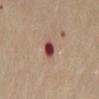This lesion was catalogued during total-body skin photography and was not selected for biopsy. A region of skin cropped from a whole-body photographic capture, roughly 15 mm wide. Automated image analysis of the tile measured a lesion-detection confidence of about 100/100. A female subject, aged 58 to 62. From the abdomen.